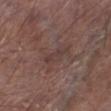Imaged during a routine full-body skin examination; the lesion was not biopsied and no histopathology is available. The recorded lesion diameter is about 3.5 mm. A 15 mm close-up tile from a total-body photography series done for melanoma screening. Imaged with white-light lighting. A male subject, about 80 years old. The lesion is on the left lower leg.